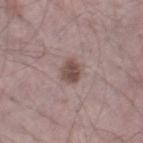The lesion was photographed on a routine skin check and not biopsied; there is no pathology result.
Automated image analysis of the tile measured a lesion–skin lightness drop of about 12 and a lesion-to-skin contrast of about 8.5 (normalized; higher = more distinct). It also reported border irregularity of about 1.5 on a 0–10 scale, a within-lesion color-variation index near 3/10, and peripheral color asymmetry of about 1.
Measured at roughly 2.5 mm in maximum diameter.
From the right lower leg.
A male subject roughly 70 years of age.
Cropped from a total-body skin-imaging series; the visible field is about 15 mm.
This is a white-light tile.